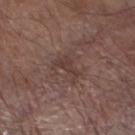Impression:
Captured during whole-body skin photography for melanoma surveillance; the lesion was not biopsied.
Image and clinical context:
This image is a 15 mm lesion crop taken from a total-body photograph. On the left lower leg. A male patient aged approximately 80.